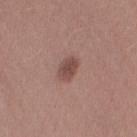Assessment: Part of a total-body skin-imaging series; this lesion was reviewed on a skin check and was not flagged for biopsy. Background: A close-up tile cropped from a whole-body skin photograph, about 15 mm across. On the arm. A female subject about 25 years old.